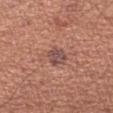Impression:
This lesion was catalogued during total-body skin photography and was not selected for biopsy.
Clinical summary:
On the left upper arm. A male subject roughly 45 years of age. This is a white-light tile. The lesion's longest dimension is about 3 mm. Cropped from a whole-body photographic skin survey; the tile spans about 15 mm. An algorithmic analysis of the crop reported an area of roughly 5 mm², an outline eccentricity of about 0.65 (0 = round, 1 = elongated), and two-axis asymmetry of about 0.2. The software also gave a within-lesion color-variation index near 3/10 and radial color variation of about 1.